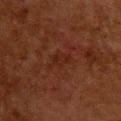Imaged during a routine full-body skin examination; the lesion was not biopsied and no histopathology is available.
The lesion is located on the upper back.
The lesion's longest dimension is about 3 mm.
A lesion tile, about 15 mm wide, cut from a 3D total-body photograph.
The subject is a female aged around 50.
The tile uses cross-polarized illumination.